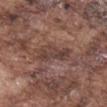Assessment: The lesion was photographed on a routine skin check and not biopsied; there is no pathology result. Background: This image is a 15 mm lesion crop taken from a total-body photograph. The lesion-visualizer software estimated a mean CIELAB color near L≈41 a*≈17 b*≈22 and about 8 CIELAB-L* units darker than the surrounding skin. The analysis additionally found a classifier nevus-likeness of about 0/100 and lesion-presence confidence of about 60/100. Measured at roughly 5 mm in maximum diameter. The tile uses white-light illumination. Located on the mid back. A male subject roughly 75 years of age.